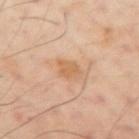notes = catalogued during a skin exam; not biopsied
image source = ~15 mm tile from a whole-body skin photo
patient = male, aged approximately 50
location = the left arm